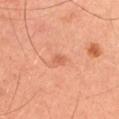– notes: no biopsy performed (imaged during a skin exam)
– TBP lesion metrics: lesion-presence confidence of about 100/100
– size: ≈1.5 mm
– location: the right upper arm
– image: ~15 mm crop, total-body skin-cancer survey
– patient: male, in their mid-40s
– tile lighting: cross-polarized illumination